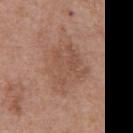Case summary:
* biopsy status: imaged on a skin check; not biopsied
* patient: male, roughly 70 years of age
* imaging modality: 15 mm crop, total-body photography
* location: the chest
* automated metrics: a lesion color around L≈51 a*≈20 b*≈28 in CIELAB and a normalized lesion–skin contrast near 5
* lighting: white-light
* size: about 6 mm